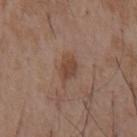Captured during whole-body skin photography for melanoma surveillance; the lesion was not biopsied. Longest diameter approximately 2.5 mm. A 15 mm crop from a total-body photograph taken for skin-cancer surveillance. A male patient, aged 53–57. This is a white-light tile. The lesion is on the abdomen.